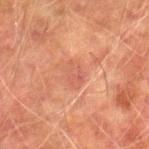Imaged during a routine full-body skin examination; the lesion was not biopsied and no histopathology is available.
Measured at roughly 3 mm in maximum diameter.
The lesion-visualizer software estimated a footprint of about 5 mm² and an eccentricity of roughly 0.7. The software also gave a border-irregularity rating of about 1.5/10 and a within-lesion color-variation index near 3/10.
The tile uses cross-polarized illumination.
The patient is a male approximately 75 years of age.
The lesion is on the right lower leg.
This image is a 15 mm lesion crop taken from a total-body photograph.